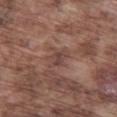The lesion was photographed on a routine skin check and not biopsied; there is no pathology result. The lesion-visualizer software estimated a footprint of about 4.5 mm² and two-axis asymmetry of about 0.35. The analysis additionally found a classifier nevus-likeness of about 0/100 and a detector confidence of about 95 out of 100 that the crop contains a lesion. This is a white-light tile. A male patient, aged 73 to 77. The lesion is located on the leg. A 15 mm close-up extracted from a 3D total-body photography capture. The lesion's longest dimension is about 3 mm.